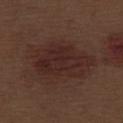biopsy_status: not biopsied; imaged during a skin examination
lighting: white-light
image:
  source: total-body photography crop
  field_of_view_mm: 15
site: right thigh
lesion_size:
  long_diameter_mm_approx: 7.5
patient:
  sex: male
  age_approx: 70